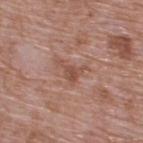illumination = white-light illumination
lesion size = about 3.5 mm
image = ~15 mm tile from a whole-body skin photo
automated lesion analysis = a footprint of about 4 mm² and two-axis asymmetry of about 0.65; a mean CIELAB color near L≈50 a*≈22 b*≈27, roughly 9 lightness units darker than nearby skin, and a normalized border contrast of about 6.5
location = the back
patient = male, approximately 70 years of age